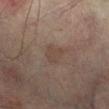Clinical impression:
This lesion was catalogued during total-body skin photography and was not selected for biopsy.
Background:
The recorded lesion diameter is about 2.5 mm. A 15 mm close-up tile from a total-body photography series done for melanoma screening. On the right lower leg. A male patient, in their mid- to late 70s.